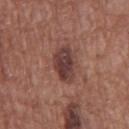Clinical impression:
The lesion was photographed on a routine skin check and not biopsied; there is no pathology result.
Context:
The patient is a male approximately 75 years of age. Approximately 4 mm at its widest. This image is a 15 mm lesion crop taken from a total-body photograph. Imaged with white-light lighting. The lesion-visualizer software estimated a border-irregularity index near 2/10 and internal color variation of about 4.5 on a 0–10 scale. The lesion is on the front of the torso.